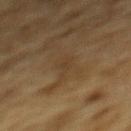Notes:
• biopsy status — no biopsy performed (imaged during a skin exam)
• lighting — cross-polarized illumination
• TBP lesion metrics — a footprint of about 3.5 mm² and an outline eccentricity of about 0.9 (0 = round, 1 = elongated); an average lesion color of about L≈32 a*≈12 b*≈26 (CIELAB), roughly 4 lightness units darker than nearby skin, and a normalized border contrast of about 4.5
• image — ~15 mm tile from a whole-body skin photo
• subject — male, in their mid- to late 80s or older
• location — the mid back